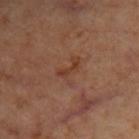This lesion was catalogued during total-body skin photography and was not selected for biopsy. The subject is a female in their mid- to late 40s. Approximately 3 mm at its widest. From the upper back. An algorithmic analysis of the crop reported a classifier nevus-likeness of about 0/100 and lesion-presence confidence of about 100/100. Cropped from a whole-body photographic skin survey; the tile spans about 15 mm. Imaged with cross-polarized lighting.